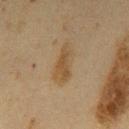<lesion>
<biopsy_status>not biopsied; imaged during a skin examination</biopsy_status>
<site>mid back</site>
<patient>
  <sex>male</sex>
  <age_approx>60</age_approx>
</patient>
<image>
  <source>total-body photography crop</source>
  <field_of_view_mm>15</field_of_view_mm>
</image>
<lesion_size>
  <long_diameter_mm_approx>5.5</long_diameter_mm_approx>
</lesion_size>
</lesion>